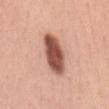Q: Was this lesion biopsied?
A: imaged on a skin check; not biopsied
Q: Where on the body is the lesion?
A: the mid back
Q: What is the lesion's diameter?
A: ≈6 mm
Q: Illumination type?
A: white-light
Q: Patient demographics?
A: female, aged 33–37
Q: What is the imaging modality?
A: total-body-photography crop, ~15 mm field of view